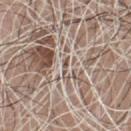Impression:
The lesion was photographed on a routine skin check and not biopsied; there is no pathology result.
Background:
Automated image analysis of the tile measured an area of roughly 1 mm² and two-axis asymmetry of about 0.35. The software also gave a mean CIELAB color near L≈54 a*≈14 b*≈23, a lesion–skin lightness drop of about 10, and a normalized border contrast of about 7. The software also gave a border-irregularity rating of about 3.5/10, internal color variation of about 0 on a 0–10 scale, and peripheral color asymmetry of about 0. It also reported a detector confidence of about 0 out of 100 that the crop contains a lesion. A region of skin cropped from a whole-body photographic capture, roughly 15 mm wide. The lesion is located on the chest. A male patient, aged around 65. Captured under white-light illumination. The lesion's longest dimension is about 2 mm.